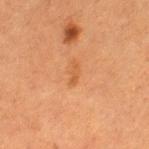The lesion was tiled from a total-body skin photograph and was not biopsied. Captured under cross-polarized illumination. Measured at roughly 2.5 mm in maximum diameter. Located on the left thigh. A female subject, in their 50s. A roughly 15 mm field-of-view crop from a total-body skin photograph.